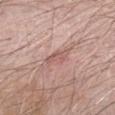No biopsy was performed on this lesion — it was imaged during a full skin examination and was not determined to be concerning.
Automated image analysis of the tile measured an area of roughly 4 mm² and an eccentricity of roughly 0.8. The analysis additionally found an average lesion color of about L≈57 a*≈21 b*≈24 (CIELAB), a lesion–skin lightness drop of about 8, and a normalized border contrast of about 5.5.
The recorded lesion diameter is about 3 mm.
On the left forearm.
A male patient roughly 65 years of age.
A lesion tile, about 15 mm wide, cut from a 3D total-body photograph.